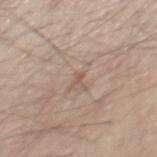Q: Was this lesion biopsied?
A: imaged on a skin check; not biopsied
Q: How was this image acquired?
A: 15 mm crop, total-body photography
Q: Where on the body is the lesion?
A: the mid back
Q: How was the tile lit?
A: white-light illumination
Q: Patient demographics?
A: male, aged 38 to 42
Q: What did automated image analysis measure?
A: a shape eccentricity near 0.8 and a symmetry-axis asymmetry near 0.35; roughly 8 lightness units darker than nearby skin and a normalized border contrast of about 5.5; border irregularity of about 3 on a 0–10 scale, a color-variation rating of about 0/10, and peripheral color asymmetry of about 0